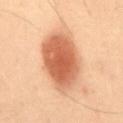- follow-up — no biopsy performed (imaged during a skin exam)
- site — the abdomen
- patient — male, about 55 years old
- image source — ~15 mm tile from a whole-body skin photo
- lesion size — ≈7 mm